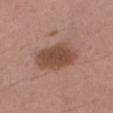Impression: Recorded during total-body skin imaging; not selected for excision or biopsy. Background: Cropped from a whole-body photographic skin survey; the tile spans about 15 mm. Automated tile analysis of the lesion measured an area of roughly 15 mm², an eccentricity of roughly 0.65, and two-axis asymmetry of about 0.1. The software also gave an average lesion color of about L≈48 a*≈21 b*≈27 (CIELAB), a lesion–skin lightness drop of about 11, and a lesion-to-skin contrast of about 8.5 (normalized; higher = more distinct). It also reported a border-irregularity rating of about 1.5/10, a color-variation rating of about 2.5/10, and peripheral color asymmetry of about 1. The analysis additionally found a lesion-detection confidence of about 100/100. The recorded lesion diameter is about 5 mm. This is a white-light tile. The subject is a male aged around 55. The lesion is located on the right upper arm.